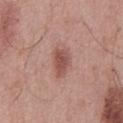The tile uses white-light illumination. An algorithmic analysis of the crop reported an area of roughly 6 mm², an eccentricity of roughly 0.85, and a symmetry-axis asymmetry near 0.25. The analysis additionally found roughly 11 lightness units darker than nearby skin and a normalized border contrast of about 7.5. The software also gave an automated nevus-likeness rating near 80 out of 100 and a lesion-detection confidence of about 100/100. A male patient approximately 55 years of age. The lesion's longest dimension is about 3.5 mm. The lesion is on the mid back. This image is a 15 mm lesion crop taken from a total-body photograph.